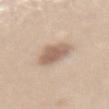follow-up — catalogued during a skin exam; not biopsied | lesion diameter — about 4 mm | anatomic site — the lower back | tile lighting — white-light illumination | automated lesion analysis — a nevus-likeness score of about 65/100 and lesion-presence confidence of about 100/100 | subject — female, aged approximately 40 | image source — ~15 mm crop, total-body skin-cancer survey.